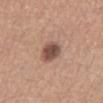Q: Was this lesion biopsied?
A: catalogued during a skin exam; not biopsied
Q: Automated lesion metrics?
A: a footprint of about 7 mm², an eccentricity of roughly 0.6, and two-axis asymmetry of about 0.25; an average lesion color of about L≈50 a*≈19 b*≈25 (CIELAB), about 14 CIELAB-L* units darker than the surrounding skin, and a lesion-to-skin contrast of about 10 (normalized; higher = more distinct); border irregularity of about 2 on a 0–10 scale, a within-lesion color-variation index near 4.5/10, and peripheral color asymmetry of about 1.5; an automated nevus-likeness rating near 80 out of 100 and lesion-presence confidence of about 100/100
Q: What is the lesion's diameter?
A: ≈3.5 mm
Q: What lighting was used for the tile?
A: white-light
Q: Patient demographics?
A: female, approximately 55 years of age
Q: What kind of image is this?
A: ~15 mm tile from a whole-body skin photo
Q: What is the anatomic site?
A: the abdomen